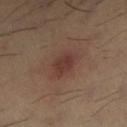This lesion was catalogued during total-body skin photography and was not selected for biopsy. Automated tile analysis of the lesion measured a nevus-likeness score of about 60/100 and lesion-presence confidence of about 100/100. Located on the left lower leg. A close-up tile cropped from a whole-body skin photograph, about 15 mm across. A male patient, aged approximately 30.